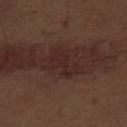notes: catalogued during a skin exam; not biopsied
anatomic site: the abdomen
tile lighting: white-light
subject: male, roughly 70 years of age
lesion diameter: ~5 mm (longest diameter)
imaging modality: 15 mm crop, total-body photography
TBP lesion metrics: a lesion area of about 10 mm² and two-axis asymmetry of about 0.35; a lesion color around L≈25 a*≈18 b*≈19 in CIELAB; a border-irregularity index near 5/10 and a color-variation rating of about 1.5/10